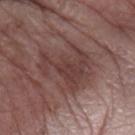Assessment:
Captured during whole-body skin photography for melanoma surveillance; the lesion was not biopsied.
Background:
The tile uses white-light illumination. A 15 mm close-up extracted from a 3D total-body photography capture. From the left forearm. A female patient in their mid- to late 80s or older. The total-body-photography lesion software estimated an area of roughly 17 mm², a shape eccentricity near 0.7, and two-axis asymmetry of about 0.6. And it measured border irregularity of about 9.5 on a 0–10 scale and peripheral color asymmetry of about 1.